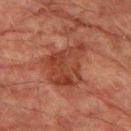Findings:
• image — ~15 mm tile from a whole-body skin photo
• lighting — cross-polarized illumination
• automated metrics — a lesion color around L≈35 a*≈24 b*≈27 in CIELAB, roughly 7 lightness units darker than nearby skin, and a lesion-to-skin contrast of about 7 (normalized; higher = more distinct)
• size — ≈6 mm
• patient — male, aged 73 to 77
• anatomic site — the left thigh